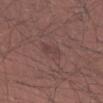| feature | finding |
|---|---|
| acquisition | ~15 mm tile from a whole-body skin photo |
| patient | male, about 30 years old |
| lesion size | ≈2.5 mm |
| illumination | white-light illumination |
| automated metrics | an average lesion color of about L≈41 a*≈19 b*≈18 (CIELAB), a lesion–skin lightness drop of about 5, and a lesion-to-skin contrast of about 4.5 (normalized; higher = more distinct); a border-irregularity index near 3/10, a within-lesion color-variation index near 1.5/10, and radial color variation of about 0.5; lesion-presence confidence of about 100/100 |
| site | the left forearm |